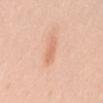  biopsy_status: not biopsied; imaged during a skin examination
  site: mid back
  lighting: white-light
  automated_metrics:
    color_variation_0_10: 1.0
    peripheral_color_asymmetry: 0.5
    lesion_detection_confidence_0_100: 100
  image:
    source: total-body photography crop
    field_of_view_mm: 15
  patient:
    sex: female
    age_approx: 40